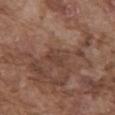| field | value |
|---|---|
| notes | catalogued during a skin exam; not biopsied |
| acquisition | 15 mm crop, total-body photography |
| lesion size | ~3 mm (longest diameter) |
| patient | male, approximately 75 years of age |
| site | the abdomen |